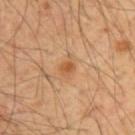<lesion>
  <biopsy_status>not biopsied; imaged during a skin examination</biopsy_status>
  <patient>
    <sex>male</sex>
    <age_approx>55</age_approx>
  </patient>
  <site>back</site>
  <image>
    <source>total-body photography crop</source>
    <field_of_view_mm>15</field_of_view_mm>
  </image>
</lesion>